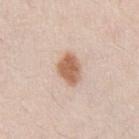biopsy_status: not biopsied; imaged during a skin examination
image:
  source: total-body photography crop
  field_of_view_mm: 15
site: abdomen
patient:
  sex: male
  age_approx: 35